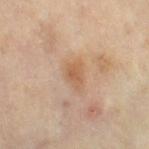The lesion was tiled from a total-body skin photograph and was not biopsied. Captured under cross-polarized illumination. A roughly 15 mm field-of-view crop from a total-body skin photograph. An algorithmic analysis of the crop reported a lesion area of about 4.5 mm², an eccentricity of roughly 0.75, and two-axis asymmetry of about 0.3. It also reported an average lesion color of about L≈56 a*≈19 b*≈33 (CIELAB), a lesion–skin lightness drop of about 8, and a normalized border contrast of about 6.5. On the leg. The patient is a female in their 50s.